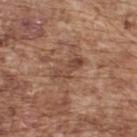Clinical impression: This lesion was catalogued during total-body skin photography and was not selected for biopsy. Clinical summary: On the upper back. Captured under white-light illumination. A region of skin cropped from a whole-body photographic capture, roughly 15 mm wide. A male patient, in their mid-70s.